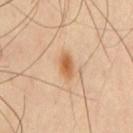Q: Was a biopsy performed?
A: imaged on a skin check; not biopsied
Q: What are the patient's age and sex?
A: male, approximately 45 years of age
Q: Automated lesion metrics?
A: a lesion area of about 5 mm², an outline eccentricity of about 0.85 (0 = round, 1 = elongated), and a symmetry-axis asymmetry near 0.15; border irregularity of about 1.5 on a 0–10 scale and a peripheral color-asymmetry measure near 1
Q: What lighting was used for the tile?
A: cross-polarized illumination
Q: What is the anatomic site?
A: the chest
Q: How was this image acquired?
A: 15 mm crop, total-body photography